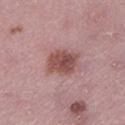This lesion was catalogued during total-body skin photography and was not selected for biopsy. A female subject roughly 45 years of age. Captured under white-light illumination. About 4 mm across. Located on the leg. A lesion tile, about 15 mm wide, cut from a 3D total-body photograph. Automated tile analysis of the lesion measured a lesion color around L≈50 a*≈24 b*≈23 in CIELAB and a normalized border contrast of about 9. And it measured a classifier nevus-likeness of about 80/100 and lesion-presence confidence of about 100/100.